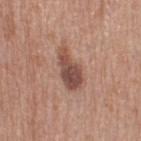– follow-up — total-body-photography surveillance lesion; no biopsy
– automated lesion analysis — a footprint of about 9 mm², a shape eccentricity near 0.9, and a symmetry-axis asymmetry near 0.3; a color-variation rating of about 4/10 and peripheral color asymmetry of about 1.5; a classifier nevus-likeness of about 60/100 and a lesion-detection confidence of about 100/100
– site — the left thigh
– image — ~15 mm crop, total-body skin-cancer survey
– illumination — white-light
– patient — female, aged around 40